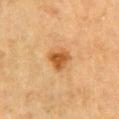• notes — catalogued during a skin exam; not biopsied
• image source — ~15 mm crop, total-body skin-cancer survey
• patient — male, aged 83 or older
• location — the right upper arm
• lighting — cross-polarized illumination
• size — ~3 mm (longest diameter)